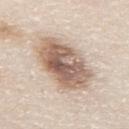Q: Lesion location?
A: the back
Q: What are the patient's age and sex?
A: male, aged around 80
Q: What is the imaging modality?
A: ~15 mm crop, total-body skin-cancer survey
Q: What lighting was used for the tile?
A: white-light
Q: Automated lesion metrics?
A: a border-irregularity rating of about 2/10, a within-lesion color-variation index near 8.5/10, and radial color variation of about 3
Q: Lesion size?
A: ~7.5 mm (longest diameter)